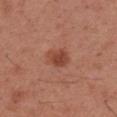Q: Was this lesion biopsied?
A: catalogued during a skin exam; not biopsied
Q: What is the lesion's diameter?
A: ≈3 mm
Q: What is the imaging modality?
A: ~15 mm tile from a whole-body skin photo
Q: Where on the body is the lesion?
A: the right forearm
Q: Patient demographics?
A: female, about 40 years old